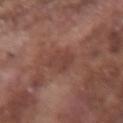The lesion was tiled from a total-body skin photograph and was not biopsied. The tile uses white-light illumination. A male patient, roughly 75 years of age. Approximately 3.5 mm at its widest. This image is a 15 mm lesion crop taken from a total-body photograph. From the right forearm.